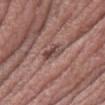lighting: white-light | anatomic site: the right thigh | image source: ~15 mm tile from a whole-body skin photo | diameter: about 3 mm | patient: female, aged around 40.